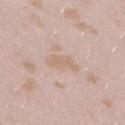Notes:
– subject · female, aged around 25
– anatomic site · the right thigh
– acquisition · ~15 mm tile from a whole-body skin photo
– diameter · about 3.5 mm
– TBP lesion metrics · a lesion-to-skin contrast of about 5 (normalized; higher = more distinct); border irregularity of about 3 on a 0–10 scale and a within-lesion color-variation index near 2/10; a classifier nevus-likeness of about 0/100 and lesion-presence confidence of about 100/100
– illumination · white-light illumination